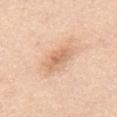<record>
  <lighting>white-light</lighting>
  <patient>
    <sex>male</sex>
    <age_approx>45</age_approx>
  </patient>
  <site>chest</site>
  <lesion_size>
    <long_diameter_mm_approx>3.5</long_diameter_mm_approx>
  </lesion_size>
  <image>
    <source>total-body photography crop</source>
    <field_of_view_mm>15</field_of_view_mm>
  </image>
</record>